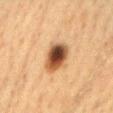Notes:
• notes · total-body-photography surveillance lesion; no biopsy
• lighting · cross-polarized illumination
• imaging modality · ~15 mm crop, total-body skin-cancer survey
• subject · female, approximately 55 years of age
• site · the chest
• lesion size · ≈4 mm